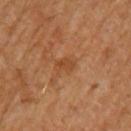Clinical impression:
The lesion was tiled from a total-body skin photograph and was not biopsied.
Image and clinical context:
Captured under cross-polarized illumination. The lesion's longest dimension is about 2.5 mm. A male patient roughly 65 years of age. On the left upper arm. The total-body-photography lesion software estimated roughly 7 lightness units darker than nearby skin and a normalized lesion–skin contrast near 6. It also reported border irregularity of about 3.5 on a 0–10 scale, a within-lesion color-variation index near 0.5/10, and peripheral color asymmetry of about 0. The software also gave a lesion-detection confidence of about 100/100. This image is a 15 mm lesion crop taken from a total-body photograph.